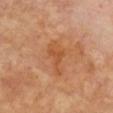Part of a total-body skin-imaging series; this lesion was reviewed on a skin check and was not flagged for biopsy.
The lesion is located on the back.
A region of skin cropped from a whole-body photographic capture, roughly 15 mm wide.
The patient is a female approximately 75 years of age.